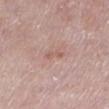body site: the left lower leg | patient: female, aged 38 to 42 | image: 15 mm crop, total-body photography | illumination: white-light illumination | automated metrics: a mean CIELAB color near L≈58 a*≈21 b*≈25, roughly 7 lightness units darker than nearby skin, and a lesion-to-skin contrast of about 5 (normalized; higher = more distinct); a nevus-likeness score of about 0/100.